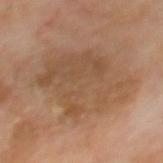Findings:
– imaging modality: total-body-photography crop, ~15 mm field of view
– location: the back
– tile lighting: cross-polarized
– size: about 8 mm
– patient: male, aged 68–72